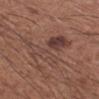Q: What did automated image analysis measure?
A: an area of roughly 16 mm², an eccentricity of roughly 0.55, and two-axis asymmetry of about 0.6
Q: Where on the body is the lesion?
A: the right forearm
Q: Lesion size?
A: ≈6.5 mm
Q: What kind of image is this?
A: ~15 mm crop, total-body skin-cancer survey
Q: Patient demographics?
A: male, about 65 years old